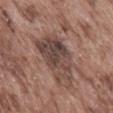biopsy status — imaged on a skin check; not biopsied
patient — male, aged approximately 75
image — ~15 mm tile from a whole-body skin photo
body site — the abdomen